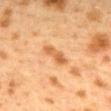notes: catalogued during a skin exam; not biopsied
patient: female, roughly 40 years of age
automated lesion analysis: internal color variation of about 3 on a 0–10 scale and a peripheral color-asymmetry measure near 1; an automated nevus-likeness rating near 5 out of 100 and a lesion-detection confidence of about 100/100
lesion size: ~3.5 mm (longest diameter)
acquisition: 15 mm crop, total-body photography
location: the mid back